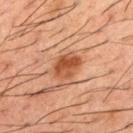workup=no biopsy performed (imaged during a skin exam)
patient=male, aged 48–52
acquisition=total-body-photography crop, ~15 mm field of view
anatomic site=the mid back
lighting=cross-polarized
size=~3.5 mm (longest diameter)